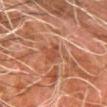Assessment: Imaged during a routine full-body skin examination; the lesion was not biopsied and no histopathology is available. Acquisition and patient details: A close-up tile cropped from a whole-body skin photograph, about 15 mm across. A male subject, aged approximately 80. The lesion is on the left forearm. The total-body-photography lesion software estimated a within-lesion color-variation index near 1/10 and a peripheral color-asymmetry measure near 0. And it measured a classifier nevus-likeness of about 0/100. This is a cross-polarized tile.